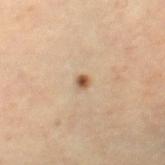Captured during whole-body skin photography for melanoma surveillance; the lesion was not biopsied.
A roughly 15 mm field-of-view crop from a total-body skin photograph.
On the right thigh.
A male subject in their mid- to late 50s.